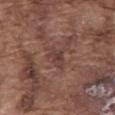biopsy_status: not biopsied; imaged during a skin examination
lesion_size:
  long_diameter_mm_approx: 2.5
site: front of the torso
image:
  source: total-body photography crop
  field_of_view_mm: 15
lighting: white-light
patient:
  sex: male
  age_approx: 75